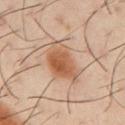Imaged during a routine full-body skin examination; the lesion was not biopsied and no histopathology is available. The subject is a male aged around 40. The tile uses cross-polarized illumination. The lesion is located on the right upper arm. A lesion tile, about 15 mm wide, cut from a 3D total-body photograph. Automated image analysis of the tile measured an area of roughly 14 mm², an outline eccentricity of about 0.7 (0 = round, 1 = elongated), and a symmetry-axis asymmetry near 0.3. It also reported about 11 CIELAB-L* units darker than the surrounding skin and a lesion-to-skin contrast of about 8.5 (normalized; higher = more distinct).